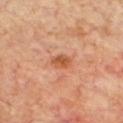Assessment:
The lesion was tiled from a total-body skin photograph and was not biopsied.
Image and clinical context:
Located on the chest. Cropped from a whole-body photographic skin survey; the tile spans about 15 mm. A male subject, aged around 70.